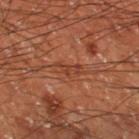biopsy_status: not biopsied; imaged during a skin examination
patient:
  sex: male
  age_approx: 60
image:
  source: total-body photography crop
  field_of_view_mm: 15
site: left thigh